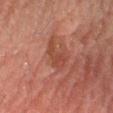No biopsy was performed on this lesion — it was imaged during a full skin examination and was not determined to be concerning.
Imaged with cross-polarized lighting.
Longest diameter approximately 3.5 mm.
On the leg.
A female subject, aged approximately 60.
A region of skin cropped from a whole-body photographic capture, roughly 15 mm wide.
Automated image analysis of the tile measured a shape eccentricity near 0.85 and two-axis asymmetry of about 0.4. It also reported a lesion color around L≈36 a*≈22 b*≈26 in CIELAB and a normalized border contrast of about 6. The analysis additionally found internal color variation of about 1 on a 0–10 scale and peripheral color asymmetry of about 0.5. And it measured an automated nevus-likeness rating near 0 out of 100 and a detector confidence of about 100 out of 100 that the crop contains a lesion.